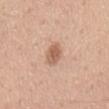{"image": {"source": "total-body photography crop", "field_of_view_mm": 15}, "site": "mid back", "lesion_size": {"long_diameter_mm_approx": 3.0}, "patient": {"sex": "male", "age_approx": 55}, "lighting": "white-light"}